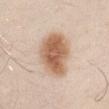Case summary:
• subject — male, in their 30s
• site — the right upper arm
• lesion size — about 6 mm
• acquisition — 15 mm crop, total-body photography
• tile lighting — white-light